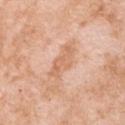This lesion was catalogued during total-body skin photography and was not selected for biopsy. A female subject in their 40s. Located on the upper back. Captured under white-light illumination. About 4 mm across. A close-up tile cropped from a whole-body skin photograph, about 15 mm across.